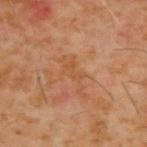notes = imaged on a skin check; not biopsied | patient = male, aged around 60 | site = the upper back | image = ~15 mm tile from a whole-body skin photo.